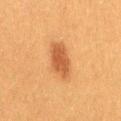Assessment:
Part of a total-body skin-imaging series; this lesion was reviewed on a skin check and was not flagged for biopsy.
Acquisition and patient details:
A 15 mm crop from a total-body photograph taken for skin-cancer surveillance. An algorithmic analysis of the crop reported an area of roughly 9 mm², an outline eccentricity of about 0.75 (0 = round, 1 = elongated), and a shape-asymmetry score of about 0.2 (0 = symmetric). Measured at roughly 4 mm in maximum diameter. This is a cross-polarized tile. The patient is a female aged around 40. The lesion is on the right thigh.